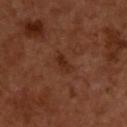Captured during whole-body skin photography for melanoma surveillance; the lesion was not biopsied. The lesion is located on the back. The tile uses cross-polarized illumination. A roughly 15 mm field-of-view crop from a total-body skin photograph. About 2.5 mm across.